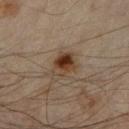Captured during whole-body skin photography for melanoma surveillance; the lesion was not biopsied. Imaged with cross-polarized lighting. A 15 mm close-up tile from a total-body photography series done for melanoma screening. Located on the right forearm. A male patient in their mid- to late 40s. The total-body-photography lesion software estimated a footprint of about 6.5 mm², a shape eccentricity near 0.55, and a shape-asymmetry score of about 0.2 (0 = symmetric).